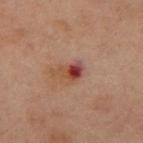{"biopsy_status": "not biopsied; imaged during a skin examination", "patient": {"sex": "male", "age_approx": 60}, "image": {"source": "total-body photography crop", "field_of_view_mm": 15}, "site": "chest", "lighting": "cross-polarized"}